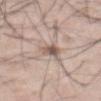{
  "biopsy_status": "not biopsied; imaged during a skin examination",
  "image": {
    "source": "total-body photography crop",
    "field_of_view_mm": 15
  },
  "patient": {
    "sex": "male",
    "age_approx": 65
  },
  "site": "mid back",
  "lesion_size": {
    "long_diameter_mm_approx": 3.0
  },
  "lighting": "white-light"
}